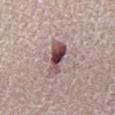Imaged during a routine full-body skin examination; the lesion was not biopsied and no histopathology is available.
Located on the right lower leg.
About 4.5 mm across.
A male subject, about 65 years old.
A lesion tile, about 15 mm wide, cut from a 3D total-body photograph.